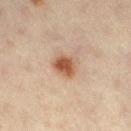Assessment: The lesion was tiled from a total-body skin photograph and was not biopsied. Image and clinical context: Captured under cross-polarized illumination. Longest diameter approximately 3 mm. A 15 mm crop from a total-body photograph taken for skin-cancer surveillance. On the leg. A female subject in their mid-40s.